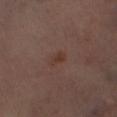notes = total-body-photography surveillance lesion; no biopsy | automated lesion analysis = a footprint of about 3 mm², an eccentricity of roughly 0.8, and two-axis asymmetry of about 0.35; a classifier nevus-likeness of about 10/100 and lesion-presence confidence of about 100/100 | image = total-body-photography crop, ~15 mm field of view | body site = the left lower leg | size = ~2.5 mm (longest diameter) | tile lighting = cross-polarized illumination.